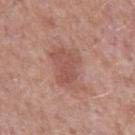biopsy status=no biopsy performed (imaged during a skin exam)
TBP lesion metrics=a footprint of about 15 mm² and a symmetry-axis asymmetry near 0.35; a classifier nevus-likeness of about 30/100 and a lesion-detection confidence of about 100/100
tile lighting=white-light
subject=male, aged 53 to 57
imaging modality=15 mm crop, total-body photography
body site=the left upper arm